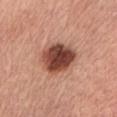lighting: white-light; subject: male, roughly 75 years of age; anatomic site: the left upper arm; diameter: ≈4.5 mm; imaging modality: total-body-photography crop, ~15 mm field of view.